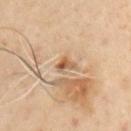Findings:
- notes — no biopsy performed (imaged during a skin exam)
- diameter — about 2.5 mm
- body site — the front of the torso
- patient — male, aged 48–52
- tile lighting — cross-polarized illumination
- acquisition — 15 mm crop, total-body photography